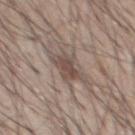Assessment: Part of a total-body skin-imaging series; this lesion was reviewed on a skin check and was not flagged for biopsy. Acquisition and patient details: Imaged with white-light lighting. A lesion tile, about 15 mm wide, cut from a 3D total-body photograph. On the chest. Measured at roughly 3.5 mm in maximum diameter. The patient is a male roughly 55 years of age. The total-body-photography lesion software estimated a lesion area of about 7.5 mm², an outline eccentricity of about 0.75 (0 = round, 1 = elongated), and a symmetry-axis asymmetry near 0.25. And it measured about 10 CIELAB-L* units darker than the surrounding skin and a lesion-to-skin contrast of about 7.5 (normalized; higher = more distinct). The analysis additionally found border irregularity of about 4 on a 0–10 scale and peripheral color asymmetry of about 1.